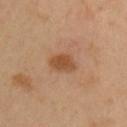| key | value |
|---|---|
| biopsy status | catalogued during a skin exam; not biopsied |
| body site | the chest |
| subject | male, about 40 years old |
| illumination | cross-polarized |
| image | total-body-photography crop, ~15 mm field of view |